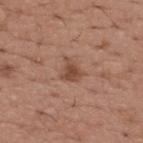– follow-up · imaged on a skin check; not biopsied
– location · the upper back
– patient · male, aged 63 to 67
– lesion diameter · ≈3 mm
– image · ~15 mm crop, total-body skin-cancer survey
– illumination · white-light illumination